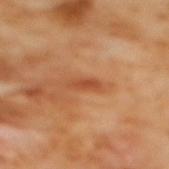Captured under cross-polarized illumination.
On the mid back.
A female subject aged around 55.
The recorded lesion diameter is about 3 mm.
A region of skin cropped from a whole-body photographic capture, roughly 15 mm wide.